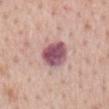Cropped from a total-body skin-imaging series; the visible field is about 15 mm. The subject is a female roughly 65 years of age. This is a white-light tile. The recorded lesion diameter is about 3.5 mm. The lesion-visualizer software estimated internal color variation of about 6 on a 0–10 scale and radial color variation of about 1.5. On the mid back.